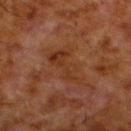This lesion was catalogued during total-body skin photography and was not selected for biopsy. The recorded lesion diameter is about 5.5 mm. The subject is a male approximately 80 years of age. The lesion-visualizer software estimated an eccentricity of roughly 0.9 and a shape-asymmetry score of about 0.45 (0 = symmetric). It also reported about 5 CIELAB-L* units darker than the surrounding skin and a normalized border contrast of about 6. The analysis additionally found a nevus-likeness score of about 0/100 and a lesion-detection confidence of about 100/100. Imaged with cross-polarized lighting. From the left lower leg. A region of skin cropped from a whole-body photographic capture, roughly 15 mm wide.